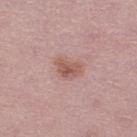workup: no biopsy performed (imaged during a skin exam)
subject: female, roughly 45 years of age
site: the right lower leg
illumination: white-light illumination
image source: ~15 mm crop, total-body skin-cancer survey
diameter: about 3.5 mm